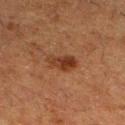This lesion was catalogued during total-body skin photography and was not selected for biopsy.
The subject is a female aged around 50.
A 15 mm crop from a total-body photograph taken for skin-cancer surveillance.
An algorithmic analysis of the crop reported a footprint of about 6 mm² and a symmetry-axis asymmetry near 0.2. The software also gave a mean CIELAB color near L≈31 a*≈21 b*≈28. And it measured peripheral color asymmetry of about 2.
Approximately 3.5 mm at its widest.
The lesion is located on the left lower leg.